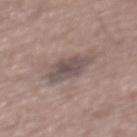{
  "biopsy_status": "not biopsied; imaged during a skin examination",
  "patient": {
    "sex": "male",
    "age_approx": 60
  },
  "lighting": "white-light",
  "site": "upper back",
  "automated_metrics": {
    "vs_skin_darker_L": 9.0,
    "vs_skin_contrast_norm": 7.5
  },
  "lesion_size": {
    "long_diameter_mm_approx": 4.5
  },
  "image": {
    "source": "total-body photography crop",
    "field_of_view_mm": 15
  }
}